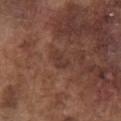Captured during whole-body skin photography for melanoma surveillance; the lesion was not biopsied.